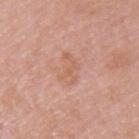This lesion was catalogued during total-body skin photography and was not selected for biopsy. Located on the left upper arm. A 15 mm close-up tile from a total-body photography series done for melanoma screening. Measured at roughly 3.5 mm in maximum diameter. A male patient, aged around 50. Imaged with white-light lighting.